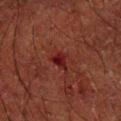Notes:
- biopsy status · catalogued during a skin exam; not biopsied
- image · ~15 mm crop, total-body skin-cancer survey
- subject · male, aged 48–52
- body site · the right forearm
- illumination · cross-polarized
- size · about 2.5 mm